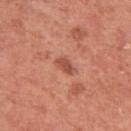Imaged during a routine full-body skin examination; the lesion was not biopsied and no histopathology is available.
A 15 mm close-up tile from a total-body photography series done for melanoma screening.
A female patient approximately 50 years of age.
From the left upper arm.
This is a white-light tile.